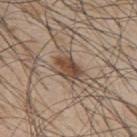<tbp_lesion>
<biopsy_status>not biopsied; imaged during a skin examination</biopsy_status>
<patient>
  <sex>male</sex>
  <age_approx>45</age_approx>
</patient>
<site>upper back</site>
<lesion_size>
  <long_diameter_mm_approx>3.5</long_diameter_mm_approx>
</lesion_size>
<image>
  <source>total-body photography crop</source>
  <field_of_view_mm>15</field_of_view_mm>
</image>
</tbp_lesion>